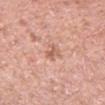follow-up: catalogued during a skin exam; not biopsied | lighting: white-light illumination | anatomic site: the arm | size: ~2.5 mm (longest diameter) | subject: female, aged approximately 40 | imaging modality: 15 mm crop, total-body photography.